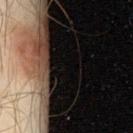follow-up=no biopsy performed (imaged during a skin exam) | lighting=cross-polarized illumination | subject=male, aged 43–47 | acquisition=15 mm crop, total-body photography | lesion size=about 10 mm | site=the left thigh.